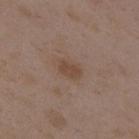– diameter · ~3 mm (longest diameter)
– patient · female, about 35 years old
– image-analysis metrics · a border-irregularity rating of about 2/10 and peripheral color asymmetry of about 0.5; a nevus-likeness score of about 5/100 and lesion-presence confidence of about 100/100
– location · the back
– imaging modality · total-body-photography crop, ~15 mm field of view
– illumination · white-light illumination